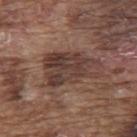Clinical impression: Imaged during a routine full-body skin examination; the lesion was not biopsied and no histopathology is available. Image and clinical context: The subject is a male aged around 75. Located on the upper back. The tile uses white-light illumination. Approximately 6.5 mm at its widest. This image is a 15 mm lesion crop taken from a total-body photograph. The total-body-photography lesion software estimated a lesion area of about 19 mm². It also reported a border-irregularity rating of about 4.5/10, a color-variation rating of about 5.5/10, and a peripheral color-asymmetry measure near 2. It also reported a nevus-likeness score of about 0/100 and a detector confidence of about 95 out of 100 that the crop contains a lesion.